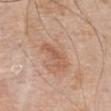  biopsy_status: not biopsied; imaged during a skin examination